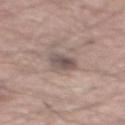The lesion was photographed on a routine skin check and not biopsied; there is no pathology result.
Located on the mid back.
A male patient, in their mid- to late 50s.
Cropped from a total-body skin-imaging series; the visible field is about 15 mm.
The tile uses white-light illumination.
Measured at roughly 3 mm in maximum diameter.